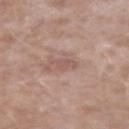A male patient aged 43–47.
The tile uses white-light illumination.
Approximately 2.5 mm at its widest.
The lesion is on the left upper arm.
A 15 mm close-up extracted from a 3D total-body photography capture.